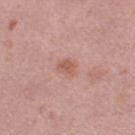Acquisition and patient details: A 15 mm close-up extracted from a 3D total-body photography capture. Located on the right thigh. Approximately 2.5 mm at its widest. The total-body-photography lesion software estimated an area of roughly 5 mm² and a symmetry-axis asymmetry near 0.2. And it measured a border-irregularity rating of about 2/10, a within-lesion color-variation index near 2.5/10, and a peripheral color-asymmetry measure near 1. And it measured an automated nevus-likeness rating near 10 out of 100. The subject is a female in their 40s.